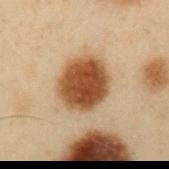{
  "biopsy_status": "not biopsied; imaged during a skin examination",
  "image": {
    "source": "total-body photography crop",
    "field_of_view_mm": 15
  },
  "lighting": "cross-polarized",
  "automated_metrics": {
    "area_mm2_approx": 20.0,
    "shape_asymmetry": 0.1,
    "cielab_L": 40,
    "cielab_a": 18,
    "cielab_b": 31,
    "vs_skin_darker_L": 16.0,
    "nevus_likeness_0_100": 100,
    "lesion_detection_confidence_0_100": 100
  },
  "site": "left upper arm",
  "patient": {
    "sex": "male",
    "age_approx": 55
  }
}